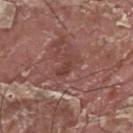No biopsy was performed on this lesion — it was imaged during a full skin examination and was not determined to be concerning. A 15 mm crop from a total-body photograph taken for skin-cancer surveillance. On the upper back. An algorithmic analysis of the crop reported a footprint of about 3.5 mm², an outline eccentricity of about 0.9 (0 = round, 1 = elongated), and two-axis asymmetry of about 0.4. And it measured a lesion-to-skin contrast of about 6 (normalized; higher = more distinct). The software also gave an automated nevus-likeness rating near 0 out of 100 and a lesion-detection confidence of about 50/100. The subject is a male in their 40s.